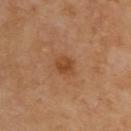Captured during whole-body skin photography for melanoma surveillance; the lesion was not biopsied. This is a cross-polarized tile. From the upper back. A region of skin cropped from a whole-body photographic capture, roughly 15 mm wide.